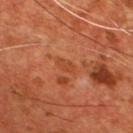- follow-up · imaged on a skin check; not biopsied
- patient · male, aged approximately 55
- location · the chest
- tile lighting · cross-polarized illumination
- imaging modality · ~15 mm tile from a whole-body skin photo